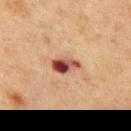– follow-up · no biopsy performed (imaged during a skin exam)
– automated metrics · an outline eccentricity of about 0.8 (0 = round, 1 = elongated); an average lesion color of about L≈39 a*≈22 b*≈24 (CIELAB), roughly 15 lightness units darker than nearby skin, and a lesion-to-skin contrast of about 12.5 (normalized; higher = more distinct)
– image source · ~15 mm tile from a whole-body skin photo
– diameter · ≈3.5 mm
– subject · male, roughly 75 years of age
– body site · the chest